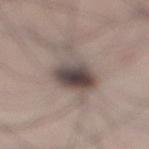{
  "biopsy_status": "not biopsied; imaged during a skin examination",
  "patient": {
    "sex": "male",
    "age_approx": 30
  },
  "lighting": "white-light",
  "image": {
    "source": "total-body photography crop",
    "field_of_view_mm": 15
  },
  "lesion_size": {
    "long_diameter_mm_approx": 7.0
  },
  "site": "right lower leg",
  "automated_metrics": {
    "area_mm2_approx": 16.0,
    "eccentricity": 0.85,
    "shape_asymmetry": 0.3,
    "cielab_L": 48,
    "cielab_a": 11,
    "cielab_b": 17,
    "vs_skin_contrast_norm": 10.0
  }
}